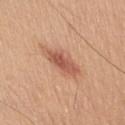Captured during whole-body skin photography for melanoma surveillance; the lesion was not biopsied. The recorded lesion diameter is about 5.5 mm. A close-up tile cropped from a whole-body skin photograph, about 15 mm across. A male subject, aged 58 to 62. On the chest. This is a white-light tile.